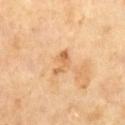Part of a total-body skin-imaging series; this lesion was reviewed on a skin check and was not flagged for biopsy. Captured under cross-polarized illumination. The lesion's longest dimension is about 3.5 mm. A 15 mm crop from a total-body photograph taken for skin-cancer surveillance. A male patient, about 65 years old.